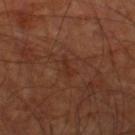Notes:
– body site — the right thigh
– imaging modality — ~15 mm tile from a whole-body skin photo
– patient — male, aged 58–62